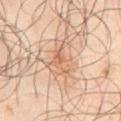Impression: No biopsy was performed on this lesion — it was imaged during a full skin examination and was not determined to be concerning. Context: On the front of the torso. Approximately 3.5 mm at its widest. A 15 mm close-up tile from a total-body photography series done for melanoma screening. The patient is a male aged approximately 65. This is a cross-polarized tile.